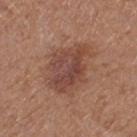workup: no biopsy performed (imaged during a skin exam)
location: the left thigh
lighting: white-light illumination
automated lesion analysis: an area of roughly 19 mm², a shape eccentricity near 0.75, and a shape-asymmetry score of about 0.25 (0 = symmetric); an average lesion color of about L≈45 a*≈22 b*≈26 (CIELAB), about 9 CIELAB-L* units darker than the surrounding skin, and a normalized lesion–skin contrast near 7
subject: female, aged 38 to 42
image: ~15 mm crop, total-body skin-cancer survey
size: ≈6 mm